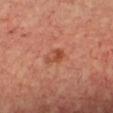Case summary:
- biopsy status — no biopsy performed (imaged during a skin exam)
- subject — female, aged 43–47
- TBP lesion metrics — an eccentricity of roughly 0.8 and two-axis asymmetry of about 0.5; a nevus-likeness score of about 40/100
- imaging modality — total-body-photography crop, ~15 mm field of view
- body site — the chest
- lighting — cross-polarized illumination